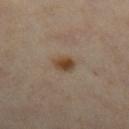| key | value |
|---|---|
| notes | imaged on a skin check; not biopsied |
| site | the right lower leg |
| illumination | cross-polarized illumination |
| imaging modality | ~15 mm tile from a whole-body skin photo |
| automated lesion analysis | an eccentricity of roughly 0.7 and a shape-asymmetry score of about 0.2 (0 = symmetric); a nevus-likeness score of about 95/100 |
| subject | female, in their mid-30s |